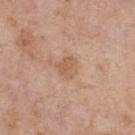Impression: Captured during whole-body skin photography for melanoma surveillance; the lesion was not biopsied. Acquisition and patient details: The patient is a male about 55 years old. The lesion-visualizer software estimated an area of roughly 4.5 mm², an outline eccentricity of about 0.35 (0 = round, 1 = elongated), and a shape-asymmetry score of about 0.35 (0 = symmetric). The analysis additionally found an automated nevus-likeness rating near 0 out of 100. A region of skin cropped from a whole-body photographic capture, roughly 15 mm wide. Approximately 2.5 mm at its widest. This is a white-light tile. Located on the upper back.